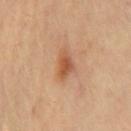workup=total-body-photography surveillance lesion; no biopsy | imaging modality=15 mm crop, total-body photography | body site=the chest | image-analysis metrics=an area of roughly 5.5 mm², a shape eccentricity near 0.8, and a symmetry-axis asymmetry near 0.35; a classifier nevus-likeness of about 80/100 and lesion-presence confidence of about 100/100 | illumination=cross-polarized illumination | size=about 3.5 mm.